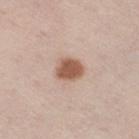  biopsy_status: not biopsied; imaged during a skin examination
  lighting: white-light
  image:
    source: total-body photography crop
    field_of_view_mm: 15
  patient:
    sex: male
    age_approx: 60
  site: right thigh
  lesion_size:
    long_diameter_mm_approx: 3.5